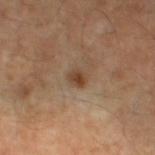Impression:
Imaged during a routine full-body skin examination; the lesion was not biopsied and no histopathology is available.
Acquisition and patient details:
Imaged with cross-polarized lighting. Located on the left thigh. An algorithmic analysis of the crop reported a border-irregularity index near 1.5/10, a color-variation rating of about 3.5/10, and peripheral color asymmetry of about 1. A close-up tile cropped from a whole-body skin photograph, about 15 mm across. Approximately 2 mm at its widest. The subject is a male aged approximately 55.